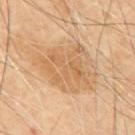<lesion>
  <biopsy_status>not biopsied; imaged during a skin examination</biopsy_status>
  <lesion_size>
    <long_diameter_mm_approx>8.0</long_diameter_mm_approx>
  </lesion_size>
  <image>
    <source>total-body photography crop</source>
    <field_of_view_mm>15</field_of_view_mm>
  </image>
  <patient>
    <sex>male</sex>
    <age_approx>70</age_approx>
  </patient>
  <site>mid back</site>
  <automated_metrics>
    <area_mm2_approx>31.0</area_mm2_approx>
    <eccentricity>0.7</eccentricity>
    <border_irregularity_0_10>3.0</border_irregularity_0_10>
    <color_variation_0_10>4.5</color_variation_0_10>
    <peripheral_color_asymmetry>1.5</peripheral_color_asymmetry>
  </automated_metrics>
</lesion>